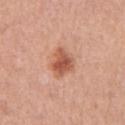biopsy status: total-body-photography surveillance lesion; no biopsy | tile lighting: white-light illumination | diameter: ≈4 mm | automated metrics: a lesion area of about 8 mm², a shape eccentricity near 0.7, and a shape-asymmetry score of about 0.3 (0 = symmetric); a border-irregularity rating of about 3/10, internal color variation of about 4 on a 0–10 scale, and radial color variation of about 1 | image: 15 mm crop, total-body photography | site: the left upper arm | subject: female, aged 58 to 62.